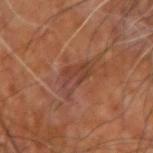Impression:
Captured during whole-body skin photography for melanoma surveillance; the lesion was not biopsied.
Context:
Longest diameter approximately 5 mm. A roughly 15 mm field-of-view crop from a total-body skin photograph. The patient is about 65 years old. The lesion is on the arm. This is a cross-polarized tile.